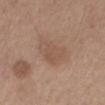workup = imaged on a skin check; not biopsied
lesion size = about 4 mm
patient = male, aged approximately 55
location = the mid back
illumination = white-light illumination
TBP lesion metrics = a footprint of about 10 mm²; a border-irregularity rating of about 4/10, a color-variation rating of about 2/10, and peripheral color asymmetry of about 0.5
image = ~15 mm crop, total-body skin-cancer survey